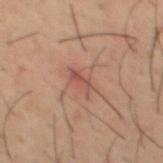Imaged during a routine full-body skin examination; the lesion was not biopsied and no histopathology is available.
The lesion is located on the mid back.
Approximately 3 mm at its widest.
The tile uses cross-polarized illumination.
A male subject about 40 years old.
A lesion tile, about 15 mm wide, cut from a 3D total-body photograph.